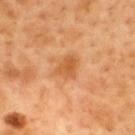Part of a total-body skin-imaging series; this lesion was reviewed on a skin check and was not flagged for biopsy.
From the upper back.
Imaged with cross-polarized lighting.
A 15 mm crop from a total-body photograph taken for skin-cancer surveillance.
A male patient, aged around 50.